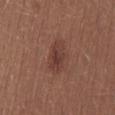Notes:
- workup · catalogued during a skin exam; not biopsied
- TBP lesion metrics · a mean CIELAB color near L≈39 a*≈21 b*≈24 and a lesion–skin lightness drop of about 8; border irregularity of about 2 on a 0–10 scale, a color-variation rating of about 3.5/10, and peripheral color asymmetry of about 1; an automated nevus-likeness rating near 30 out of 100
- lesion size · about 4.5 mm
- image source · 15 mm crop, total-body photography
- anatomic site · the mid back
- patient · female, aged 23 to 27
- tile lighting · white-light illumination